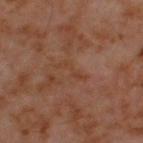The lesion was tiled from a total-body skin photograph and was not biopsied. The lesion-visualizer software estimated a shape eccentricity near 0.95. The lesion is on the upper back. The patient is a male about 60 years old. A 15 mm close-up extracted from a 3D total-body photography capture. Approximately 3.5 mm at its widest.